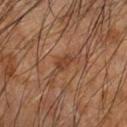notes: no biopsy performed (imaged during a skin exam); subject: male, aged approximately 60; size: ~3 mm (longest diameter); tile lighting: cross-polarized illumination; body site: the left forearm; acquisition: 15 mm crop, total-body photography.